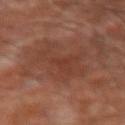Impression:
Imaged during a routine full-body skin examination; the lesion was not biopsied and no histopathology is available.
Clinical summary:
On the right forearm. Imaged with cross-polarized lighting. The recorded lesion diameter is about 8 mm. This image is a 15 mm lesion crop taken from a total-body photograph. An algorithmic analysis of the crop reported a footprint of about 19 mm², an eccentricity of roughly 0.9, and two-axis asymmetry of about 0.5. And it measured border irregularity of about 8 on a 0–10 scale, a color-variation rating of about 2.5/10, and peripheral color asymmetry of about 1. And it measured a classifier nevus-likeness of about 0/100 and a detector confidence of about 75 out of 100 that the crop contains a lesion. A male subject in their mid- to late 60s.